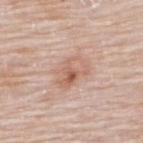{"biopsy_status": "not biopsied; imaged during a skin examination", "image": {"source": "total-body photography crop", "field_of_view_mm": 15}, "lesion_size": {"long_diameter_mm_approx": 4.5}, "site": "upper back", "automated_metrics": {"area_mm2_approx": 11.0, "eccentricity": 0.75, "shape_asymmetry": 0.2, "cielab_L": 62, "cielab_a": 20, "cielab_b": 28, "vs_skin_darker_L": 8.0, "vs_skin_contrast_norm": 6.0, "border_irregularity_0_10": 2.5, "color_variation_0_10": 8.0, "peripheral_color_asymmetry": 3.0, "nevus_likeness_0_100": 15, "lesion_detection_confidence_0_100": 100}, "lighting": "white-light", "patient": {"sex": "male", "age_approx": 75}}